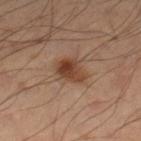Located on the left leg. The patient is a male in their 50s. A 15 mm close-up extracted from a 3D total-body photography capture. Approximately 3.5 mm at its widest.